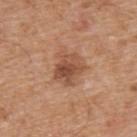Captured during whole-body skin photography for melanoma surveillance; the lesion was not biopsied.
Cropped from a whole-body photographic skin survey; the tile spans about 15 mm.
A male patient, in their mid-60s.
Captured under white-light illumination.
Automated image analysis of the tile measured a lesion area of about 10 mm², an outline eccentricity of about 0.45 (0 = round, 1 = elongated), and a symmetry-axis asymmetry near 0.25. The analysis additionally found a lesion color around L≈51 a*≈22 b*≈32 in CIELAB.
Longest diameter approximately 4 mm.
The lesion is on the upper back.